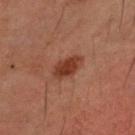biopsy status: imaged on a skin check; not biopsied | patient: male, aged 48 to 52 | tile lighting: cross-polarized illumination | image source: ~15 mm tile from a whole-body skin photo | site: the head or neck | diameter: ≈3 mm.